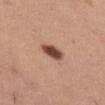<case>
<biopsy_status>not biopsied; imaged during a skin examination</biopsy_status>
<image>
  <source>total-body photography crop</source>
  <field_of_view_mm>15</field_of_view_mm>
</image>
<lighting>white-light</lighting>
<lesion_size>
  <long_diameter_mm_approx>3.5</long_diameter_mm_approx>
</lesion_size>
<patient>
  <sex>female</sex>
  <age_approx>30</age_approx>
</patient>
<automated_metrics>
  <vs_skin_darker_L>18.0</vs_skin_darker_L>
  <vs_skin_contrast_norm>12.5</vs_skin_contrast_norm>
</automated_metrics>
<site>abdomen</site>
</case>